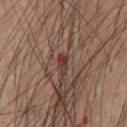* image source: total-body-photography crop, ~15 mm field of view
* patient: male, approximately 60 years of age
* body site: the chest